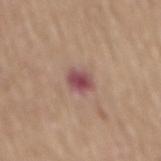No biopsy was performed on this lesion — it was imaged during a full skin examination and was not determined to be concerning.
This is a white-light tile.
Measured at roughly 3 mm in maximum diameter.
From the mid back.
The subject is a male aged approximately 70.
A 15 mm crop from a total-body photograph taken for skin-cancer surveillance.